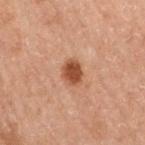Impression: Imaged during a routine full-body skin examination; the lesion was not biopsied and no histopathology is available. Background: A 15 mm close-up tile from a total-body photography series done for melanoma screening. This is a cross-polarized tile. The lesion is located on the leg. A male subject, in their 70s. Longest diameter approximately 3 mm.